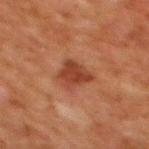Imaged during a routine full-body skin examination; the lesion was not biopsied and no histopathology is available. A male subject aged around 80. The lesion's longest dimension is about 3.5 mm. The total-body-photography lesion software estimated a footprint of about 7.5 mm² and an outline eccentricity of about 0.65 (0 = round, 1 = elongated). And it measured a lesion-detection confidence of about 100/100. The tile uses cross-polarized illumination. A region of skin cropped from a whole-body photographic capture, roughly 15 mm wide. On the back.